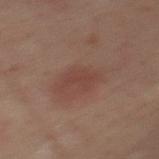– illumination · cross-polarized
– site · the back
– image source · ~15 mm crop, total-body skin-cancer survey
– subject · male, aged approximately 40
– automated lesion analysis · an eccentricity of roughly 0.6; an average lesion color of about L≈32 a*≈17 b*≈19 (CIELAB), a lesion–skin lightness drop of about 4, and a lesion-to-skin contrast of about 4.5 (normalized; higher = more distinct)
– lesion diameter · ≈2.5 mm